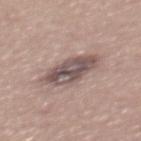- biopsy status: catalogued during a skin exam; not biopsied
- site: the back
- lesion diameter: ~5.5 mm (longest diameter)
- lighting: white-light illumination
- subject: male, aged 38 to 42
- acquisition: total-body-photography crop, ~15 mm field of view